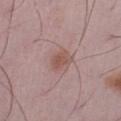  biopsy_status: not biopsied; imaged during a skin examination
  site: leg
  image:
    source: total-body photography crop
    field_of_view_mm: 15
  lesion_size:
    long_diameter_mm_approx: 3.0
  patient:
    sex: male
    age_approx: 50
  lighting: white-light